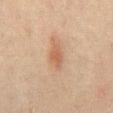biopsy status = no biopsy performed (imaged during a skin exam) | image = 15 mm crop, total-body photography | subject = male, aged 43–47 | illumination = cross-polarized | anatomic site = the abdomen | lesion diameter = about 3 mm.